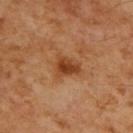{
  "biopsy_status": "not biopsied; imaged during a skin examination",
  "site": "upper back",
  "lesion_size": {
    "long_diameter_mm_approx": 3.0
  },
  "lighting": "cross-polarized",
  "patient": {
    "sex": "male",
    "age_approx": 60
  },
  "image": {
    "source": "total-body photography crop",
    "field_of_view_mm": 15
  }
}